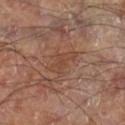Recorded during total-body skin imaging; not selected for excision or biopsy. Automated image analysis of the tile measured a lesion area of about 6 mm², a shape eccentricity near 0.8, and two-axis asymmetry of about 0.5. And it measured roughly 5 lightness units darker than nearby skin and a lesion-to-skin contrast of about 5 (normalized; higher = more distinct). The software also gave a border-irregularity index near 6/10, a within-lesion color-variation index near 1/10, and radial color variation of about 0.5. From the right lower leg. A male patient, in their 70s. A 15 mm close-up extracted from a 3D total-body photography capture. This is a cross-polarized tile. Measured at roughly 3.5 mm in maximum diameter.